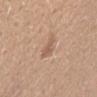Clinical impression: Recorded during total-body skin imaging; not selected for excision or biopsy. Image and clinical context: A 15 mm crop from a total-body photograph taken for skin-cancer surveillance. Imaged with white-light lighting. A female patient aged 48–52. Measured at roughly 2.5 mm in maximum diameter. From the lower back.